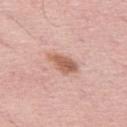Imaged during a routine full-body skin examination; the lesion was not biopsied and no histopathology is available.
The lesion is on the left thigh.
A lesion tile, about 15 mm wide, cut from a 3D total-body photograph.
A female patient in their mid- to late 60s.